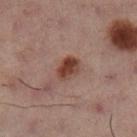Part of a total-body skin-imaging series; this lesion was reviewed on a skin check and was not flagged for biopsy. A region of skin cropped from a whole-body photographic capture, roughly 15 mm wide. A female patient aged around 55. The lesion is located on the left leg.